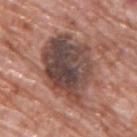No biopsy was performed on this lesion — it was imaged during a full skin examination and was not determined to be concerning. A male patient, aged around 70. Cropped from a total-body skin-imaging series; the visible field is about 15 mm. Captured under white-light illumination. The lesion is located on the upper back. Approximately 8 mm at its widest.